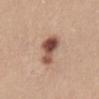<record>
  <biopsy_status>not biopsied; imaged during a skin examination</biopsy_status>
  <patient>
    <sex>female</sex>
    <age_approx>25</age_approx>
  </patient>
  <site>abdomen</site>
  <image>
    <source>total-body photography crop</source>
    <field_of_view_mm>15</field_of_view_mm>
  </image>
  <automated_metrics>
    <cielab_L>52</cielab_L>
    <cielab_a>21</cielab_a>
    <cielab_b>27</cielab_b>
    <vs_skin_darker_L>16.0</vs_skin_darker_L>
    <vs_skin_contrast_norm>10.5</vs_skin_contrast_norm>
    <peripheral_color_asymmetry>3.0</peripheral_color_asymmetry>
    <nevus_likeness_0_100>95</nevus_likeness_0_100>
    <lesion_detection_confidence_0_100>100</lesion_detection_confidence_0_100>
  </automated_metrics>
  <lighting>white-light</lighting>
</record>